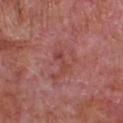follow-up: no biopsy performed (imaged during a skin exam)
body site: the front of the torso
illumination: white-light
automated metrics: a footprint of about 3.5 mm² and an outline eccentricity of about 0.9 (0 = round, 1 = elongated); a lesion color around L≈45 a*≈28 b*≈27 in CIELAB, about 6 CIELAB-L* units darker than the surrounding skin, and a lesion-to-skin contrast of about 5.5 (normalized; higher = more distinct); a border-irregularity index near 4/10, a color-variation rating of about 2.5/10, and peripheral color asymmetry of about 0.5; a classifier nevus-likeness of about 0/100 and a lesion-detection confidence of about 100/100
lesion size: ≈3 mm
patient: male, aged 63–67
image source: ~15 mm tile from a whole-body skin photo